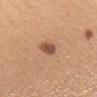The lesion was photographed on a routine skin check and not biopsied; there is no pathology result. An algorithmic analysis of the crop reported about 13 CIELAB-L* units darker than the surrounding skin and a lesion-to-skin contrast of about 9 (normalized; higher = more distinct). And it measured a border-irregularity index near 3/10 and radial color variation of about 0.5. The analysis additionally found a classifier nevus-likeness of about 95/100. About 3.5 mm across. A female patient aged around 30. The tile uses white-light illumination. The lesion is located on the upper back. Cropped from a total-body skin-imaging series; the visible field is about 15 mm.